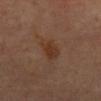biopsy_status: not biopsied; imaged during a skin examination
site: mid back
automated_metrics:
  cielab_L: 32
  cielab_a: 18
  cielab_b: 27
  vs_skin_darker_L: 6.0
  vs_skin_contrast_norm: 7.0
image:
  source: total-body photography crop
  field_of_view_mm: 15
lighting: cross-polarized
patient:
  sex: male
  age_approx: 70